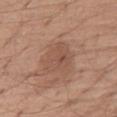{
  "biopsy_status": "not biopsied; imaged during a skin examination",
  "site": "chest",
  "image": {
    "source": "total-body photography crop",
    "field_of_view_mm": 15
  },
  "lesion_size": {
    "long_diameter_mm_approx": 4.5
  },
  "patient": {
    "sex": "male",
    "age_approx": 75
  }
}